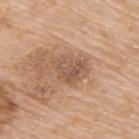The lesion was tiled from a total-body skin photograph and was not biopsied. Longest diameter approximately 4.5 mm. An algorithmic analysis of the crop reported a lesion color around L≈55 a*≈19 b*≈31 in CIELAB, a lesion–skin lightness drop of about 10, and a normalized border contrast of about 7. And it measured a border-irregularity rating of about 3.5/10 and internal color variation of about 4 on a 0–10 scale. Captured under white-light illumination. A close-up tile cropped from a whole-body skin photograph, about 15 mm across. The lesion is on the right upper arm. The patient is a female aged 73 to 77.